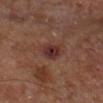Notes:
– biopsy status · total-body-photography surveillance lesion; no biopsy
– body site · the leg
– imaging modality · ~15 mm crop, total-body skin-cancer survey
– patient · aged 63 to 67